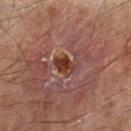Q: Was a biopsy performed?
A: total-body-photography surveillance lesion; no biopsy
Q: What is the imaging modality?
A: 15 mm crop, total-body photography
Q: Lesion size?
A: ≈3 mm
Q: What lighting was used for the tile?
A: cross-polarized
Q: What did automated image analysis measure?
A: a lesion area of about 4.5 mm², a shape eccentricity near 0.75, and a symmetry-axis asymmetry near 0.3; a detector confidence of about 100 out of 100 that the crop contains a lesion
Q: Lesion location?
A: the left forearm